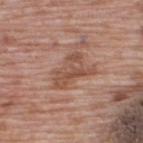{"biopsy_status": "not biopsied; imaged during a skin examination", "automated_metrics": {"border_irregularity_0_10": 6.0, "color_variation_0_10": 3.5, "peripheral_color_asymmetry": 1.5}, "image": {"source": "total-body photography crop", "field_of_view_mm": 15}, "lesion_size": {"long_diameter_mm_approx": 5.0}, "site": "upper back", "patient": {"sex": "male", "age_approx": 70}}